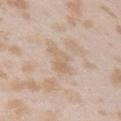Impression:
Recorded during total-body skin imaging; not selected for excision or biopsy.
Background:
Imaged with white-light lighting. Approximately 5 mm at its widest. A female subject, approximately 25 years of age. The lesion is located on the arm. A 15 mm crop from a total-body photograph taken for skin-cancer surveillance.